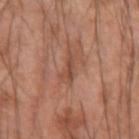Q: Was this lesion biopsied?
A: no biopsy performed (imaged during a skin exam)
Q: What kind of image is this?
A: total-body-photography crop, ~15 mm field of view
Q: What lighting was used for the tile?
A: cross-polarized illumination
Q: What is the anatomic site?
A: the left forearm
Q: Patient demographics?
A: male, aged approximately 60
Q: Automated lesion metrics?
A: a lesion area of about 3.5 mm²; border irregularity of about 5.5 on a 0–10 scale, internal color variation of about 0 on a 0–10 scale, and a peripheral color-asymmetry measure near 0; a nevus-likeness score of about 0/100 and a detector confidence of about 55 out of 100 that the crop contains a lesion